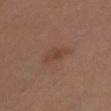Q: What are the patient's age and sex?
A: male, about 35 years old
Q: What lighting was used for the tile?
A: cross-polarized illumination
Q: What kind of image is this?
A: ~15 mm tile from a whole-body skin photo
Q: Automated lesion metrics?
A: a lesion area of about 6.5 mm² and two-axis asymmetry of about 0.3; a mean CIELAB color near L≈40 a*≈20 b*≈26, about 5 CIELAB-L* units darker than the surrounding skin, and a normalized border contrast of about 5
Q: Where on the body is the lesion?
A: the chest
Q: Lesion size?
A: ≈3.5 mm